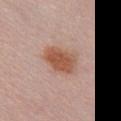The lesion is on the back. A region of skin cropped from a whole-body photographic capture, roughly 15 mm wide. The patient is a female roughly 25 years of age. Captured under white-light illumination.